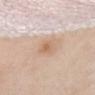Q: What did automated image analysis measure?
A: a lesion area of about 5.5 mm², an outline eccentricity of about 0.7 (0 = round, 1 = elongated), and a shape-asymmetry score of about 0.2 (0 = symmetric); about 8 CIELAB-L* units darker than the surrounding skin and a normalized lesion–skin contrast near 6.5; a color-variation rating of about 3.5/10 and a peripheral color-asymmetry measure near 1
Q: What is the anatomic site?
A: the front of the torso
Q: Who is the patient?
A: female, aged around 60
Q: How was this image acquired?
A: ~15 mm crop, total-body skin-cancer survey
Q: What is the lesion's diameter?
A: ~3 mm (longest diameter)